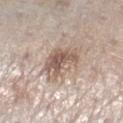Assessment: Recorded during total-body skin imaging; not selected for excision or biopsy. Acquisition and patient details: A male patient, aged around 60. Longest diameter approximately 5 mm. A region of skin cropped from a whole-body photographic capture, roughly 15 mm wide. The tile uses white-light illumination. The lesion is located on the right lower leg.